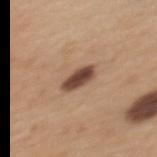Recorded during total-body skin imaging; not selected for excision or biopsy. Captured under white-light illumination. A 15 mm crop from a total-body photograph taken for skin-cancer surveillance. The total-body-photography lesion software estimated a lesion area of about 6 mm², an outline eccentricity of about 0.85 (0 = round, 1 = elongated), and a symmetry-axis asymmetry near 0.15. And it measured a border-irregularity rating of about 2/10, internal color variation of about 4 on a 0–10 scale, and a peripheral color-asymmetry measure near 1. A female patient aged approximately 30. On the upper back. Measured at roughly 4 mm in maximum diameter.